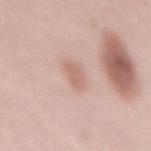Clinical impression: Part of a total-body skin-imaging series; this lesion was reviewed on a skin check and was not flagged for biopsy. Context: A close-up tile cropped from a whole-body skin photograph, about 15 mm across. The lesion-visualizer software estimated a shape-asymmetry score of about 0.15 (0 = symmetric). Longest diameter approximately 3 mm. A female patient, approximately 60 years of age. Located on the mid back. Captured under white-light illumination.